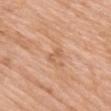This lesion was catalogued during total-body skin photography and was not selected for biopsy.